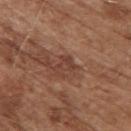Impression:
The lesion was photographed on a routine skin check and not biopsied; there is no pathology result.
Context:
The lesion's longest dimension is about 2.5 mm. On the upper back. A close-up tile cropped from a whole-body skin photograph, about 15 mm across. Captured under white-light illumination. Automated tile analysis of the lesion measured a lesion color around L≈41 a*≈22 b*≈27 in CIELAB and roughly 7 lightness units darker than nearby skin. It also reported a lesion-detection confidence of about 90/100. A male subject, aged approximately 75.